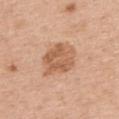Clinical impression:
The lesion was photographed on a routine skin check and not biopsied; there is no pathology result.
Image and clinical context:
A 15 mm close-up extracted from a 3D total-body photography capture. Captured under white-light illumination. The recorded lesion diameter is about 5.5 mm. Located on the upper back. The patient is a female in their mid- to late 60s.